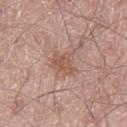| key | value |
|---|---|
| notes | catalogued during a skin exam; not biopsied |
| acquisition | ~15 mm crop, total-body skin-cancer survey |
| patient | male, roughly 55 years of age |
| site | the left thigh |
| illumination | white-light |
| lesion size | ≈3 mm |
| automated lesion analysis | a footprint of about 6.5 mm², an outline eccentricity of about 0.5 (0 = round, 1 = elongated), and two-axis asymmetry of about 0.25; a classifier nevus-likeness of about 0/100 and lesion-presence confidence of about 100/100 |